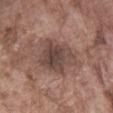No biopsy was performed on this lesion — it was imaged during a full skin examination and was not determined to be concerning.